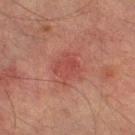{
  "biopsy_status": "not biopsied; imaged during a skin examination",
  "automated_metrics": {
    "area_mm2_approx": 9.0,
    "eccentricity": 0.45,
    "shape_asymmetry": 0.25
  },
  "patient": {
    "sex": "male",
    "age_approx": 75
  },
  "image": {
    "source": "total-body photography crop",
    "field_of_view_mm": 15
  },
  "site": "leg",
  "lesion_size": {
    "long_diameter_mm_approx": 4.0
  }
}